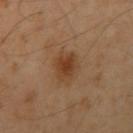Captured during whole-body skin photography for melanoma surveillance; the lesion was not biopsied.
A male patient, roughly 50 years of age.
On the right upper arm.
Imaged with cross-polarized lighting.
The recorded lesion diameter is about 3 mm.
This image is a 15 mm lesion crop taken from a total-body photograph.
The total-body-photography lesion software estimated a footprint of about 6.5 mm², a shape eccentricity near 0.4, and a symmetry-axis asymmetry near 0.25.